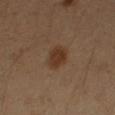The lesion was photographed on a routine skin check and not biopsied; there is no pathology result.
The subject is a male aged 48–52.
Longest diameter approximately 3 mm.
The lesion is located on the left arm.
Automated image analysis of the tile measured a footprint of about 6 mm², an eccentricity of roughly 0.65, and a symmetry-axis asymmetry near 0.2.
Cropped from a total-body skin-imaging series; the visible field is about 15 mm.
The tile uses cross-polarized illumination.